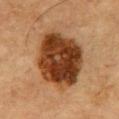  biopsy_status: not biopsied; imaged during a skin examination
  lighting: cross-polarized
  lesion_size:
    long_diameter_mm_approx: 8.0
  image:
    source: total-body photography crop
    field_of_view_mm: 15
  site: chest
  patient:
    sex: male
    age_approx: 85+